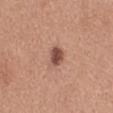image source — ~15 mm crop, total-body skin-cancer survey
size — ≈2.5 mm
subject — female, aged 28–32
automated metrics — a lesion area of about 4.5 mm² and an outline eccentricity of about 0.6 (0 = round, 1 = elongated); a lesion color around L≈50 a*≈22 b*≈28 in CIELAB, about 14 CIELAB-L* units darker than the surrounding skin, and a normalized border contrast of about 9.5
site — the upper back
illumination — white-light